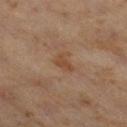size = about 2.5 mm; illumination = cross-polarized; patient = male, aged approximately 60; imaging modality = ~15 mm crop, total-body skin-cancer survey; body site = the right thigh.